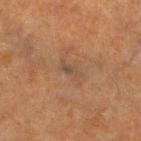Impression: Captured during whole-body skin photography for melanoma surveillance; the lesion was not biopsied. Acquisition and patient details: A male patient about 65 years old. Captured under cross-polarized illumination. Located on the right lower leg. A region of skin cropped from a whole-body photographic capture, roughly 15 mm wide.